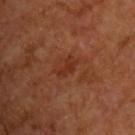follow-up: imaged on a skin check; not biopsied | automated metrics: a mean CIELAB color near L≈30 a*≈25 b*≈30 and about 6 CIELAB-L* units darker than the surrounding skin; an automated nevus-likeness rating near 0 out of 100 and lesion-presence confidence of about 100/100 | patient: male, aged around 65 | diameter: about 3 mm | lighting: cross-polarized illumination | imaging modality: ~15 mm tile from a whole-body skin photo | anatomic site: the chest.